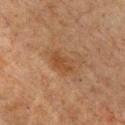Assessment:
No biopsy was performed on this lesion — it was imaged during a full skin examination and was not determined to be concerning.
Image and clinical context:
A male subject aged approximately 65. The lesion is located on the chest. A lesion tile, about 15 mm wide, cut from a 3D total-body photograph. About 3.5 mm across. Captured under cross-polarized illumination.